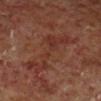Q: Is there a histopathology result?
A: no biopsy performed (imaged during a skin exam)
Q: Lesion location?
A: the right lower leg
Q: What are the patient's age and sex?
A: male, aged around 60
Q: What is the imaging modality?
A: total-body-photography crop, ~15 mm field of view